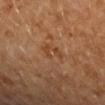Assessment:
Imaged during a routine full-body skin examination; the lesion was not biopsied and no histopathology is available.
Clinical summary:
A female patient aged around 65. The lesion is located on the left forearm. A roughly 15 mm field-of-view crop from a total-body skin photograph.